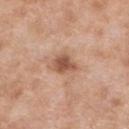Recorded during total-body skin imaging; not selected for excision or biopsy. The lesion is on the left lower leg. The recorded lesion diameter is about 3.5 mm. The subject is a male aged around 80. A 15 mm crop from a total-body photograph taken for skin-cancer surveillance.